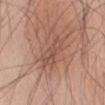Recorded during total-body skin imaging; not selected for excision or biopsy. The lesion is located on the abdomen. The patient is a male aged approximately 60. A close-up tile cropped from a whole-body skin photograph, about 15 mm across.